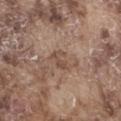The lesion was photographed on a routine skin check and not biopsied; there is no pathology result.
A roughly 15 mm field-of-view crop from a total-body skin photograph.
Located on the right thigh.
The total-body-photography lesion software estimated a footprint of about 4 mm², an outline eccentricity of about 0.75 (0 = round, 1 = elongated), and two-axis asymmetry of about 0.45. And it measured a lesion color around L≈49 a*≈17 b*≈26 in CIELAB, about 8 CIELAB-L* units darker than the surrounding skin, and a normalized border contrast of about 6. And it measured a nevus-likeness score of about 0/100 and a detector confidence of about 95 out of 100 that the crop contains a lesion.
A male patient in their mid-70s.